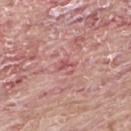automated lesion analysis = a peripheral color-asymmetry measure near 0; a classifier nevus-likeness of about 0/100 and lesion-presence confidence of about 90/100
subject = male, aged 63 to 67
anatomic site = the upper back
image source = total-body-photography crop, ~15 mm field of view
lighting = white-light illumination